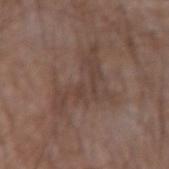follow-up: imaged on a skin check; not biopsied | body site: the left forearm | automated lesion analysis: a lesion color around L≈41 a*≈15 b*≈22 in CIELAB and a lesion–skin lightness drop of about 6; a border-irregularity rating of about 10/10 and internal color variation of about 2.5 on a 0–10 scale; a nevus-likeness score of about 0/100 | patient: male, about 55 years old | lighting: white-light illumination | imaging modality: 15 mm crop, total-body photography | diameter: ≈7 mm.